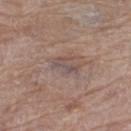Case summary:
– workup — catalogued during a skin exam; not biopsied
– site — the left thigh
– acquisition — ~15 mm tile from a whole-body skin photo
– subject — female, approximately 70 years of age